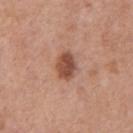Case summary:
– body site — the chest
– imaging modality — ~15 mm tile from a whole-body skin photo
– patient — male, aged 68 to 72